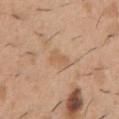biopsy status: catalogued during a skin exam; not biopsied
diameter: ≈2.5 mm
lighting: white-light illumination
anatomic site: the chest
patient: male, aged around 40
acquisition: total-body-photography crop, ~15 mm field of view